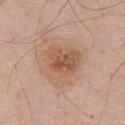<record>
  <biopsy_status>not biopsied; imaged during a skin examination</biopsy_status>
  <image>
    <source>total-body photography crop</source>
    <field_of_view_mm>15</field_of_view_mm>
  </image>
  <lesion_size>
    <long_diameter_mm_approx>5.0</long_diameter_mm_approx>
  </lesion_size>
  <patient>
    <sex>male</sex>
    <age_approx>60</age_approx>
  </patient>
  <site>chest</site>
  <lighting>white-light</lighting>
</record>